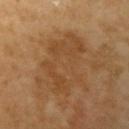| key | value |
|---|---|
| workup | catalogued during a skin exam; not biopsied |
| anatomic site | the left upper arm |
| automated lesion analysis | internal color variation of about 3.5 on a 0–10 scale and radial color variation of about 1 |
| image | 15 mm crop, total-body photography |
| lesion size | ~7.5 mm (longest diameter) |
| subject | female, aged 68 to 72 |